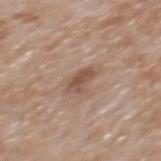Clinical impression:
The lesion was photographed on a routine skin check and not biopsied; there is no pathology result.
Clinical summary:
The total-body-photography lesion software estimated a lesion area of about 5 mm² and an eccentricity of roughly 0.8. And it measured a color-variation rating of about 2/10. On the mid back. Measured at roughly 3 mm in maximum diameter. A region of skin cropped from a whole-body photographic capture, roughly 15 mm wide. A male patient about 65 years old.